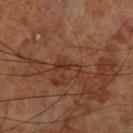Q: Was this lesion biopsied?
A: total-body-photography surveillance lesion; no biopsy
Q: What did automated image analysis measure?
A: a border-irregularity index near 6/10, a within-lesion color-variation index near 0.5/10, and radial color variation of about 0; an automated nevus-likeness rating near 0 out of 100 and a detector confidence of about 100 out of 100 that the crop contains a lesion
Q: Lesion location?
A: the right lower leg
Q: Patient demographics?
A: male, aged 68–72
Q: What lighting was used for the tile?
A: cross-polarized illumination
Q: Lesion size?
A: ~3 mm (longest diameter)
Q: How was this image acquired?
A: total-body-photography crop, ~15 mm field of view